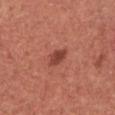Background: On the upper back. A female patient in their mid-30s. Approximately 3 mm at its widest. Imaged with white-light lighting. Cropped from a total-body skin-imaging series; the visible field is about 15 mm.